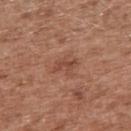Case summary:
– biopsy status · catalogued during a skin exam; not biopsied
– image source · ~15 mm crop, total-body skin-cancer survey
– subject · male, roughly 75 years of age
– tile lighting · white-light illumination
– lesion diameter · ~3 mm (longest diameter)
– site · the right upper arm